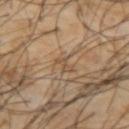No biopsy was performed on this lesion — it was imaged during a full skin examination and was not determined to be concerning.
From the left forearm.
The lesion-visualizer software estimated an outline eccentricity of about 0.9 (0 = round, 1 = elongated) and two-axis asymmetry of about 0.35. It also reported an average lesion color of about L≈51 a*≈15 b*≈32 (CIELAB), about 7 CIELAB-L* units darker than the surrounding skin, and a normalized lesion–skin contrast near 5. The software also gave a border-irregularity index near 4.5/10, internal color variation of about 0 on a 0–10 scale, and radial color variation of about 0. And it measured a nevus-likeness score of about 0/100 and lesion-presence confidence of about 20/100.
Cropped from a total-body skin-imaging series; the visible field is about 15 mm.
Longest diameter approximately 2.5 mm.
Imaged with cross-polarized lighting.
A male subject aged 38 to 42.